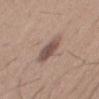<case>
  <biopsy_status>not biopsied; imaged during a skin examination</biopsy_status>
  <image>
    <source>total-body photography crop</source>
    <field_of_view_mm>15</field_of_view_mm>
  </image>
  <lesion_size>
    <long_diameter_mm_approx>4.5</long_diameter_mm_approx>
  </lesion_size>
  <patient>
    <sex>male</sex>
    <age_approx>65</age_approx>
  </patient>
  <lighting>white-light</lighting>
  <site>mid back</site>
</case>